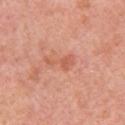biopsy_status: not biopsied; imaged during a skin examination
image:
  source: total-body photography crop
  field_of_view_mm: 15
lesion_size:
  long_diameter_mm_approx: 3.5
patient:
  sex: female
  age_approx: 55
site: left upper arm